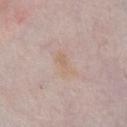Q: Was this lesion biopsied?
A: no biopsy performed (imaged during a skin exam)
Q: How large is the lesion?
A: about 3 mm
Q: Lesion location?
A: the chest
Q: How was this image acquired?
A: total-body-photography crop, ~15 mm field of view
Q: Who is the patient?
A: female, aged 63–67
Q: What lighting was used for the tile?
A: white-light